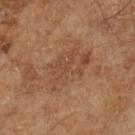Part of a total-body skin-imaging series; this lesion was reviewed on a skin check and was not flagged for biopsy. An algorithmic analysis of the crop reported an average lesion color of about L≈45 a*≈19 b*≈30 (CIELAB) and a normalized border contrast of about 5. The analysis additionally found border irregularity of about 5 on a 0–10 scale, a within-lesion color-variation index near 3.5/10, and peripheral color asymmetry of about 1.5. Imaged with cross-polarized lighting. Longest diameter approximately 6.5 mm. A male subject, aged 63–67. A lesion tile, about 15 mm wide, cut from a 3D total-body photograph.